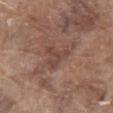Assessment:
This lesion was catalogued during total-body skin photography and was not selected for biopsy.
Background:
A male patient aged around 80. This image is a 15 mm lesion crop taken from a total-body photograph. On the chest. Measured at roughly 3.5 mm in maximum diameter. Imaged with white-light lighting.